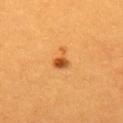Impression: Imaged during a routine full-body skin examination; the lesion was not biopsied and no histopathology is available. Image and clinical context: An algorithmic analysis of the crop reported a footprint of about 4 mm² and an outline eccentricity of about 0.65 (0 = round, 1 = elongated). The analysis additionally found a mean CIELAB color near L≈52 a*≈27 b*≈46. It also reported border irregularity of about 5 on a 0–10 scale, internal color variation of about 4.5 on a 0–10 scale, and radial color variation of about 1.5. On the back. A lesion tile, about 15 mm wide, cut from a 3D total-body photograph. A female patient about 30 years old. Captured under cross-polarized illumination.